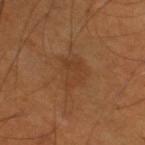Q: Was a biopsy performed?
A: no biopsy performed (imaged during a skin exam)
Q: What lighting was used for the tile?
A: cross-polarized illumination
Q: Lesion location?
A: the right thigh
Q: Patient demographics?
A: male, aged around 55
Q: What is the imaging modality?
A: ~15 mm crop, total-body skin-cancer survey